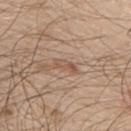This lesion was catalogued during total-body skin photography and was not selected for biopsy. The total-body-photography lesion software estimated a lesion area of about 3 mm², an eccentricity of roughly 0.9, and a shape-asymmetry score of about 0.4 (0 = symmetric). It also reported a lesion color around L≈54 a*≈17 b*≈29 in CIELAB and a normalized lesion–skin contrast near 6. It also reported a nevus-likeness score of about 0/100 and a detector confidence of about 75 out of 100 that the crop contains a lesion. A 15 mm close-up extracted from a 3D total-body photography capture. The subject is a male aged approximately 50. The lesion is on the upper back.